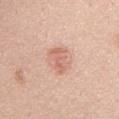Recorded during total-body skin imaging; not selected for excision or biopsy.
A female patient, aged around 40.
Imaged with white-light lighting.
From the chest.
Measured at roughly 3.5 mm in maximum diameter.
Automated image analysis of the tile measured a footprint of about 4.5 mm², an eccentricity of roughly 0.95, and a symmetry-axis asymmetry near 0.35.
A roughly 15 mm field-of-view crop from a total-body skin photograph.